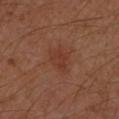No biopsy was performed on this lesion — it was imaged during a full skin examination and was not determined to be concerning.
The patient is a female aged approximately 40.
Located on the left forearm.
This image is a 15 mm lesion crop taken from a total-body photograph.